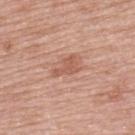workup=no biopsy performed (imaged during a skin exam)
site=the back
image-analysis metrics=a color-variation rating of about 1/10 and peripheral color asymmetry of about 0.5
imaging modality=15 mm crop, total-body photography
tile lighting=white-light
lesion diameter=≈3.5 mm
subject=female, roughly 60 years of age